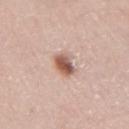– follow-up — catalogued during a skin exam; not biopsied
– acquisition — 15 mm crop, total-body photography
– lighting — white-light
– TBP lesion metrics — a footprint of about 5.5 mm² and an eccentricity of roughly 0.65
– body site — the left arm
– patient — female, in their 40s
– diameter — about 3 mm